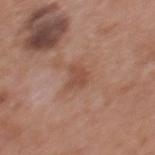* notes: catalogued during a skin exam; not biopsied
* site: the mid back
* subject: male, aged 68–72
* illumination: white-light
* acquisition: ~15 mm crop, total-body skin-cancer survey
* lesion size: ≈2.5 mm
* automated metrics: a lesion area of about 4.5 mm²; an average lesion color of about L≈49 a*≈22 b*≈29 (CIELAB), roughly 8 lightness units darker than nearby skin, and a lesion-to-skin contrast of about 6 (normalized; higher = more distinct); a border-irregularity rating of about 3.5/10, internal color variation of about 2 on a 0–10 scale, and peripheral color asymmetry of about 0.5; an automated nevus-likeness rating near 0 out of 100 and a detector confidence of about 100 out of 100 that the crop contains a lesion